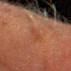<tbp_lesion>
  <biopsy_status>not biopsied; imaged during a skin examination</biopsy_status>
  <site>head or neck</site>
  <image>
    <source>total-body photography crop</source>
    <field_of_view_mm>15</field_of_view_mm>
  </image>
  <lighting>cross-polarized</lighting>
  <lesion_size>
    <long_diameter_mm_approx>4.5</long_diameter_mm_approx>
  </lesion_size>
  <patient>
    <sex>male</sex>
    <age_approx>60</age_approx>
  </patient>
  <automated_metrics>
    <eccentricity>0.95</eccentricity>
    <vs_skin_darker_L>5.0</vs_skin_darker_L>
    <vs_skin_contrast_norm>5.0</vs_skin_contrast_norm>
    <nevus_likeness_0_100>0</nevus_likeness_0_100>
    <lesion_detection_confidence_0_100>55</lesion_detection_confidence_0_100>
  </automated_metrics>
</tbp_lesion>